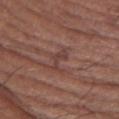{
  "biopsy_status": "not biopsied; imaged during a skin examination",
  "patient": {
    "sex": "male",
    "age_approx": 75
  },
  "lighting": "white-light",
  "image": {
    "source": "total-body photography crop",
    "field_of_view_mm": 15
  },
  "lesion_size": {
    "long_diameter_mm_approx": 3.0
  },
  "site": "right forearm"
}